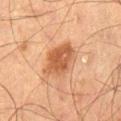Q: Was a biopsy performed?
A: imaged on a skin check; not biopsied
Q: What is the imaging modality?
A: ~15 mm crop, total-body skin-cancer survey
Q: Lesion location?
A: the leg
Q: What lighting was used for the tile?
A: cross-polarized illumination
Q: Who is the patient?
A: male, aged around 65
Q: Automated lesion metrics?
A: a border-irregularity index near 2.5/10, a color-variation rating of about 3.5/10, and a peripheral color-asymmetry measure near 1; a classifier nevus-likeness of about 90/100
Q: How large is the lesion?
A: ~4.5 mm (longest diameter)